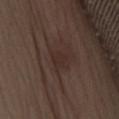Recorded during total-body skin imaging; not selected for excision or biopsy.
The total-body-photography lesion software estimated an area of roughly 3 mm² and an eccentricity of roughly 0.15. It also reported an average lesion color of about L≈27 a*≈15 b*≈19 (CIELAB), roughly 4 lightness units darker than nearby skin, and a normalized border contrast of about 5. And it measured border irregularity of about 3 on a 0–10 scale and a color-variation rating of about 1/10. The software also gave an automated nevus-likeness rating near 0 out of 100 and lesion-presence confidence of about 100/100.
This is a white-light tile.
Approximately 2 mm at its widest.
A lesion tile, about 15 mm wide, cut from a 3D total-body photograph.
Located on the chest.
A female patient aged 48 to 52.